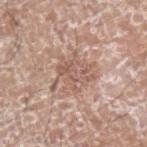notes=imaged on a skin check; not biopsied
image=15 mm crop, total-body photography
automated lesion analysis=border irregularity of about 7.5 on a 0–10 scale, internal color variation of about 4 on a 0–10 scale, and a peripheral color-asymmetry measure near 1.5; a classifier nevus-likeness of about 0/100
site=the left upper arm
lesion size=~5.5 mm (longest diameter)
illumination=white-light
patient=male, in their mid-70s